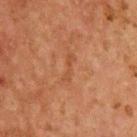• workup · total-body-photography surveillance lesion; no biopsy
• lesion size · ~3.5 mm (longest diameter)
• imaging modality · ~15 mm crop, total-body skin-cancer survey
• site · the chest
• subject · male, aged around 65
• automated metrics · a lesion area of about 3 mm², a shape eccentricity near 0.95, and a shape-asymmetry score of about 0.45 (0 = symmetric); an average lesion color of about L≈39 a*≈22 b*≈31 (CIELAB) and roughly 5 lightness units darker than nearby skin; border irregularity of about 7 on a 0–10 scale; a classifier nevus-likeness of about 0/100